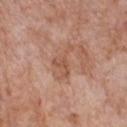<lesion>
<patient>
  <sex>female</sex>
  <age_approx>70</age_approx>
</patient>
<lighting>white-light</lighting>
<lesion_size>
  <long_diameter_mm_approx>4.0</long_diameter_mm_approx>
</lesion_size>
<site>chest</site>
<image>
  <source>total-body photography crop</source>
  <field_of_view_mm>15</field_of_view_mm>
</image>
</lesion>